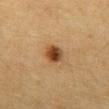The lesion was tiled from a total-body skin photograph and was not biopsied. The lesion-visualizer software estimated a footprint of about 5 mm² and an outline eccentricity of about 0.65 (0 = round, 1 = elongated). The analysis additionally found a lesion–skin lightness drop of about 14. The software also gave a border-irregularity index near 2/10, a color-variation rating of about 7/10, and peripheral color asymmetry of about 2.5. The lesion's longest dimension is about 3 mm. Cropped from a total-body skin-imaging series; the visible field is about 15 mm. The tile uses cross-polarized illumination. Located on the front of the torso. A male patient aged around 85 or older.